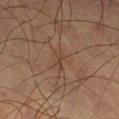{
  "lesion_size": {
    "long_diameter_mm_approx": 3.0
  },
  "patient": {
    "sex": "male",
    "age_approx": 65
  },
  "site": "leg",
  "automated_metrics": {
    "area_mm2_approx": 3.0,
    "eccentricity": 0.9,
    "shape_asymmetry": 0.4,
    "cielab_L": 32,
    "cielab_a": 14,
    "cielab_b": 22,
    "vs_skin_darker_L": 4.0,
    "vs_skin_contrast_norm": 4.5
  },
  "lighting": "cross-polarized",
  "image": {
    "source": "total-body photography crop",
    "field_of_view_mm": 15
  }
}